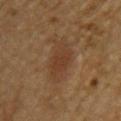  site: upper back
  lighting: cross-polarized
  lesion_size:
    long_diameter_mm_approx: 5.5
  image:
    source: total-body photography crop
    field_of_view_mm: 15
  patient:
    sex: male
    age_approx: 85+